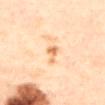Part of a total-body skin-imaging series; this lesion was reviewed on a skin check and was not flagged for biopsy.
Longest diameter approximately 3 mm.
A female subject, about 60 years old.
The lesion is located on the upper back.
The total-body-photography lesion software estimated a shape eccentricity near 0.9 and a shape-asymmetry score of about 0.55 (0 = symmetric). The analysis additionally found a lesion color around L≈74 a*≈24 b*≈43 in CIELAB, roughly 12 lightness units darker than nearby skin, and a normalized lesion–skin contrast near 7.5.
A 15 mm crop from a total-body photograph taken for skin-cancer surveillance.
Captured under cross-polarized illumination.